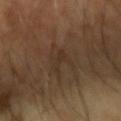Impression: Recorded during total-body skin imaging; not selected for excision or biopsy. Acquisition and patient details: A male subject, in their mid-60s. The lesion-visualizer software estimated a lesion area of about 3.5 mm², an outline eccentricity of about 0.95 (0 = round, 1 = elongated), and a symmetry-axis asymmetry near 0.55. The software also gave a mean CIELAB color near L≈30 a*≈14 b*≈24, about 5 CIELAB-L* units darker than the surrounding skin, and a lesion-to-skin contrast of about 5.5 (normalized; higher = more distinct). It also reported a border-irregularity index near 7/10 and a within-lesion color-variation index near 0/10. A 15 mm close-up tile from a total-body photography series done for melanoma screening. Imaged with cross-polarized lighting. Approximately 4 mm at its widest.